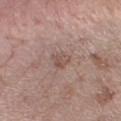A male patient aged 68–72. A close-up tile cropped from a whole-body skin photograph, about 15 mm across. From the right forearm.